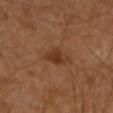Recorded during total-body skin imaging; not selected for excision or biopsy. Imaged with cross-polarized lighting. Automated tile analysis of the lesion measured a shape eccentricity near 0.75 and a shape-asymmetry score of about 0.25 (0 = symmetric). The analysis additionally found an average lesion color of about L≈34 a*≈21 b*≈31 (CIELAB) and a normalized border contrast of about 7.5. The software also gave border irregularity of about 2.5 on a 0–10 scale, a within-lesion color-variation index near 2.5/10, and a peripheral color-asymmetry measure near 1. The analysis additionally found a classifier nevus-likeness of about 70/100 and a detector confidence of about 100 out of 100 that the crop contains a lesion. A male subject, aged 83 or older. The lesion is located on the right forearm. Cropped from a whole-body photographic skin survey; the tile spans about 15 mm. About 3.5 mm across.